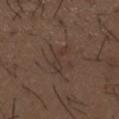Findings:
- workup · no biopsy performed (imaged during a skin exam)
- automated metrics · an outline eccentricity of about 0.9 (0 = round, 1 = elongated) and two-axis asymmetry of about 0.6; a border-irregularity rating of about 8/10 and a color-variation rating of about 0/10; lesion-presence confidence of about 100/100
- location · the mid back
- lighting · white-light
- image source · ~15 mm crop, total-body skin-cancer survey
- patient · male, aged 48–52